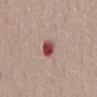biopsy_status: not biopsied; imaged during a skin examination
image:
  source: total-body photography crop
  field_of_view_mm: 15
patient:
  sex: female
  age_approx: 65
lesion_size:
  long_diameter_mm_approx: 3.0
site: back
lighting: white-light